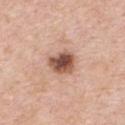Part of a total-body skin-imaging series; this lesion was reviewed on a skin check and was not flagged for biopsy.
Located on the chest.
The tile uses white-light illumination.
The subject is a male about 55 years old.
About 3.5 mm across.
Cropped from a whole-body photographic skin survey; the tile spans about 15 mm.